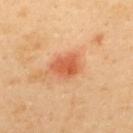follow-up=catalogued during a skin exam; not biopsied
diameter=about 3.5 mm
patient=male, roughly 40 years of age
lighting=cross-polarized
site=the upper back
imaging modality=total-body-photography crop, ~15 mm field of view